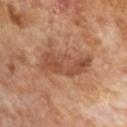Clinical impression:
Recorded during total-body skin imaging; not selected for excision or biopsy.
Clinical summary:
A male patient, aged approximately 65. A roughly 15 mm field-of-view crop from a total-body skin photograph. This is a cross-polarized tile. The total-body-photography lesion software estimated a footprint of about 16 mm², an outline eccentricity of about 0.8 (0 = round, 1 = elongated), and a symmetry-axis asymmetry near 0.4. And it measured a lesion color around L≈48 a*≈22 b*≈32 in CIELAB, roughly 9 lightness units darker than nearby skin, and a normalized border contrast of about 6.5. And it measured an automated nevus-likeness rating near 40 out of 100 and lesion-presence confidence of about 100/100. Longest diameter approximately 5 mm.